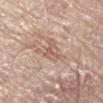No biopsy was performed on this lesion — it was imaged during a full skin examination and was not determined to be concerning.
On the right arm.
A region of skin cropped from a whole-body photographic capture, roughly 15 mm wide.
The subject is a male aged 78 to 82.